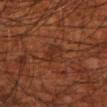The lesion was tiled from a total-body skin photograph and was not biopsied. This is a cross-polarized tile. The lesion's longest dimension is about 3.5 mm. A region of skin cropped from a whole-body photographic capture, roughly 15 mm wide. The lesion is located on the left forearm. Automated tile analysis of the lesion measured a lesion color around L≈29 a*≈23 b*≈29 in CIELAB, a lesion–skin lightness drop of about 5, and a lesion-to-skin contrast of about 5.5 (normalized; higher = more distinct). The analysis additionally found border irregularity of about 3.5 on a 0–10 scale, internal color variation of about 2 on a 0–10 scale, and radial color variation of about 0.5. The software also gave lesion-presence confidence of about 85/100. A male patient about 70 years old.